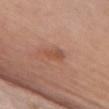Case summary:
* follow-up: total-body-photography surveillance lesion; no biopsy
* lesion size: about 2.5 mm
* TBP lesion metrics: an area of roughly 3 mm², an outline eccentricity of about 0.85 (0 = round, 1 = elongated), and a symmetry-axis asymmetry near 0.25; border irregularity of about 3 on a 0–10 scale, a color-variation rating of about 1.5/10, and a peripheral color-asymmetry measure near 0; an automated nevus-likeness rating near 5 out of 100
* lighting: white-light
* subject: female, roughly 80 years of age
* acquisition: total-body-photography crop, ~15 mm field of view
* body site: the chest